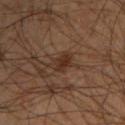Findings:
– follow-up: imaged on a skin check; not biopsied
– subject: male, roughly 55 years of age
– image: ~15 mm crop, total-body skin-cancer survey
– anatomic site: the back
– lighting: cross-polarized illumination